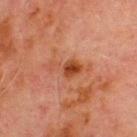Recorded during total-body skin imaging; not selected for excision or biopsy. A male patient, aged 68–72. The lesion is on the chest. This is a cross-polarized tile. A region of skin cropped from a whole-body photographic capture, roughly 15 mm wide.